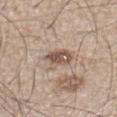{
  "biopsy_status": "not biopsied; imaged during a skin examination",
  "patient": {
    "sex": "male",
    "age_approx": 45
  },
  "site": "left lower leg",
  "image": {
    "source": "total-body photography crop",
    "field_of_view_mm": 15
  }
}